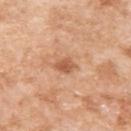Automated image analysis of the tile measured a footprint of about 4 mm², an eccentricity of roughly 0.65, and a shape-asymmetry score of about 0.2 (0 = symmetric). The analysis additionally found a border-irregularity index near 2/10, a color-variation rating of about 2.5/10, and radial color variation of about 0.5. It also reported a nevus-likeness score of about 5/100 and a lesion-detection confidence of about 100/100.
This is a white-light tile.
A region of skin cropped from a whole-body photographic capture, roughly 15 mm wide.
Longest diameter approximately 2.5 mm.
The lesion is located on the left upper arm.
A female subject, aged approximately 75.